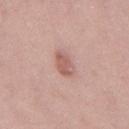Case summary:
• biopsy status: catalogued during a skin exam; not biopsied
• location: the mid back
• TBP lesion metrics: a lesion area of about 5 mm², an eccentricity of roughly 0.85, and two-axis asymmetry of about 0.25; a lesion color around L≈59 a*≈22 b*≈25 in CIELAB, roughly 10 lightness units darker than nearby skin, and a lesion-to-skin contrast of about 6.5 (normalized; higher = more distinct); border irregularity of about 2.5 on a 0–10 scale and peripheral color asymmetry of about 1; a nevus-likeness score of about 10/100 and a detector confidence of about 100 out of 100 that the crop contains a lesion
• illumination: white-light illumination
• patient: male, about 40 years old
• image: 15 mm crop, total-body photography